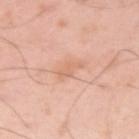The lesion was tiled from a total-body skin photograph and was not biopsied. The total-body-photography lesion software estimated an area of roughly 4 mm² and a symmetry-axis asymmetry near 0.4. It also reported a detector confidence of about 100 out of 100 that the crop contains a lesion. The lesion is on the left upper arm. The subject is a male aged around 40. Captured under white-light illumination. Measured at roughly 3 mm in maximum diameter. A close-up tile cropped from a whole-body skin photograph, about 15 mm across.